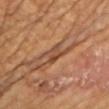Clinical impression:
The lesion was tiled from a total-body skin photograph and was not biopsied.
Image and clinical context:
The subject is a male approximately 70 years of age. An algorithmic analysis of the crop reported a lesion area of about 3.5 mm² and a shape-asymmetry score of about 0.35 (0 = symmetric). The software also gave border irregularity of about 4 on a 0–10 scale and a within-lesion color-variation index near 0/10. It also reported a classifier nevus-likeness of about 0/100. A 15 mm close-up tile from a total-body photography series done for melanoma screening. Measured at roughly 3 mm in maximum diameter. The lesion is on the chest. Captured under cross-polarized illumination.